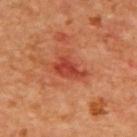No biopsy was performed on this lesion — it was imaged during a full skin examination and was not determined to be concerning. A female subject aged approximately 40. Located on the upper back. Captured under cross-polarized illumination. The recorded lesion diameter is about 3.5 mm. A roughly 15 mm field-of-view crop from a total-body skin photograph. An algorithmic analysis of the crop reported an area of roughly 6 mm², a shape eccentricity near 0.85, and a shape-asymmetry score of about 0.4 (0 = symmetric). The software also gave about 11 CIELAB-L* units darker than the surrounding skin. The software also gave a nevus-likeness score of about 5/100 and a lesion-detection confidence of about 100/100.